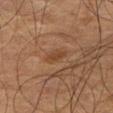| feature | finding |
|---|---|
| biopsy status | catalogued during a skin exam; not biopsied |
| lesion size | ~2.5 mm (longest diameter) |
| acquisition | 15 mm crop, total-body photography |
| tile lighting | cross-polarized illumination |
| body site | the right thigh |
| subject | male, roughly 75 years of age |